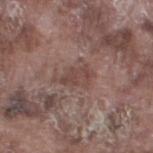Impression: The lesion was tiled from a total-body skin photograph and was not biopsied. Background: The lesion is located on the leg. A male patient in their mid-70s. Automated image analysis of the tile measured a footprint of about 6.5 mm², a shape eccentricity near 0.85, and a symmetry-axis asymmetry near 0.35. The analysis additionally found a nevus-likeness score of about 0/100. A lesion tile, about 15 mm wide, cut from a 3D total-body photograph. Captured under white-light illumination.